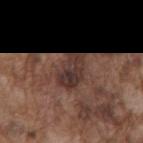Context: The patient is a male aged around 75. The lesion is located on the mid back. The total-body-photography lesion software estimated a lesion–skin lightness drop of about 9 and a normalized lesion–skin contrast near 9. And it measured a detector confidence of about 95 out of 100 that the crop contains a lesion. Imaged with white-light lighting. A lesion tile, about 15 mm wide, cut from a 3D total-body photograph. Measured at roughly 4.5 mm in maximum diameter.